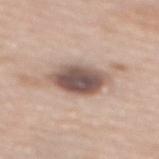Q: Was a biopsy performed?
A: no biopsy performed (imaged during a skin exam)
Q: Who is the patient?
A: female, about 60 years old
Q: What kind of image is this?
A: total-body-photography crop, ~15 mm field of view
Q: What is the anatomic site?
A: the back
Q: What did automated image analysis measure?
A: a lesion–skin lightness drop of about 18 and a normalized lesion–skin contrast near 11.5
Q: Lesion size?
A: about 6 mm